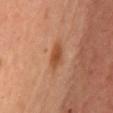Case summary:
– workup: total-body-photography surveillance lesion; no biopsy
– subject: female, aged 38–42
– lesion size: ≈3.5 mm
– acquisition: 15 mm crop, total-body photography
– location: the chest
– tile lighting: cross-polarized